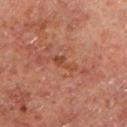Part of a total-body skin-imaging series; this lesion was reviewed on a skin check and was not flagged for biopsy.
A male subject aged 58 to 62.
Located on the left lower leg.
Imaged with cross-polarized lighting.
A roughly 15 mm field-of-view crop from a total-body skin photograph.
Approximately 3.5 mm at its widest.
An algorithmic analysis of the crop reported internal color variation of about 1 on a 0–10 scale.